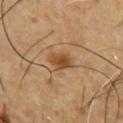notes: catalogued during a skin exam; not biopsied
automated metrics: an average lesion color of about L≈47 a*≈20 b*≈37 (CIELAB), a lesion–skin lightness drop of about 10, and a normalized lesion–skin contrast near 8
lesion diameter: ~3 mm (longest diameter)
anatomic site: the chest
illumination: cross-polarized
subject: male, approximately 55 years of age
acquisition: ~15 mm crop, total-body skin-cancer survey